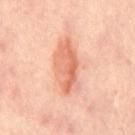{"biopsy_status": "not biopsied; imaged during a skin examination", "lesion_size": {"long_diameter_mm_approx": 7.0}, "patient": {"sex": "female", "age_approx": 50}, "site": "chest", "lighting": "cross-polarized", "image": {"source": "total-body photography crop", "field_of_view_mm": 15}}